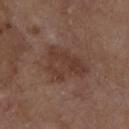workup: catalogued during a skin exam; not biopsied | size: ≈5.5 mm | subject: male, roughly 80 years of age | image: total-body-photography crop, ~15 mm field of view | illumination: white-light illumination | anatomic site: the chest.